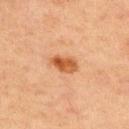- follow-up — imaged on a skin check; not biopsied
- site — the front of the torso
- size — ≈3.5 mm
- illumination — cross-polarized illumination
- image — 15 mm crop, total-body photography
- subject — male, aged 63–67
- TBP lesion metrics — a border-irregularity index near 2/10 and radial color variation of about 2